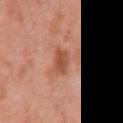Q: Is there a histopathology result?
A: total-body-photography surveillance lesion; no biopsy
Q: What lighting was used for the tile?
A: white-light
Q: What is the imaging modality?
A: ~15 mm crop, total-body skin-cancer survey
Q: What did automated image analysis measure?
A: a footprint of about 5 mm², an eccentricity of roughly 0.65, and two-axis asymmetry of about 0.2; an average lesion color of about L≈52 a*≈26 b*≈33 (CIELAB), about 11 CIELAB-L* units darker than the surrounding skin, and a lesion-to-skin contrast of about 7.5 (normalized; higher = more distinct); a border-irregularity rating of about 2/10, internal color variation of about 2.5 on a 0–10 scale, and peripheral color asymmetry of about 1; an automated nevus-likeness rating near 25 out of 100 and a detector confidence of about 100 out of 100 that the crop contains a lesion
Q: What are the patient's age and sex?
A: female, roughly 40 years of age
Q: Where on the body is the lesion?
A: the right upper arm